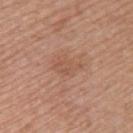Q: Is there a histopathology result?
A: imaged on a skin check; not biopsied
Q: What is the imaging modality?
A: ~15 mm tile from a whole-body skin photo
Q: What is the anatomic site?
A: the left upper arm
Q: What lighting was used for the tile?
A: white-light illumination
Q: What are the patient's age and sex?
A: female, aged around 60
Q: What did automated image analysis measure?
A: a shape eccentricity near 0.75 and a shape-asymmetry score of about 0.35 (0 = symmetric); an average lesion color of about L≈54 a*≈22 b*≈30 (CIELAB) and about 6 CIELAB-L* units darker than the surrounding skin; an automated nevus-likeness rating near 0 out of 100